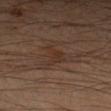biopsy status=imaged on a skin check; not biopsied
acquisition=~15 mm crop, total-body skin-cancer survey
location=the arm
subject=male, approximately 40 years of age